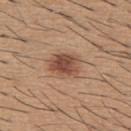Q: Was a biopsy performed?
A: catalogued during a skin exam; not biopsied
Q: What lighting was used for the tile?
A: white-light illumination
Q: Lesion size?
A: ≈3.5 mm
Q: What kind of image is this?
A: ~15 mm tile from a whole-body skin photo
Q: What are the patient's age and sex?
A: male, aged around 60
Q: Lesion location?
A: the back